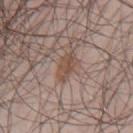Captured during whole-body skin photography for melanoma surveillance; the lesion was not biopsied. A 15 mm crop from a total-body photograph taken for skin-cancer surveillance. About 3.5 mm across. Captured under white-light illumination. On the mid back. The patient is a male in their mid- to late 40s.